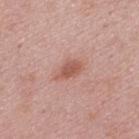Recorded during total-body skin imaging; not selected for excision or biopsy. From the back. This image is a 15 mm lesion crop taken from a total-body photograph. Imaged with white-light lighting. The subject is a male aged 43–47. Automated tile analysis of the lesion measured an average lesion color of about L≈55 a*≈25 b*≈28 (CIELAB), a lesion–skin lightness drop of about 10, and a normalized lesion–skin contrast near 7.5.